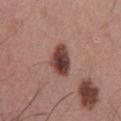Q: Is there a histopathology result?
A: catalogued during a skin exam; not biopsied
Q: What is the anatomic site?
A: the chest
Q: Patient demographics?
A: male, roughly 40 years of age
Q: What lighting was used for the tile?
A: white-light
Q: How was this image acquired?
A: ~15 mm tile from a whole-body skin photo
Q: Lesion size?
A: about 4 mm
Q: What did automated image analysis measure?
A: a mean CIELAB color near L≈41 a*≈21 b*≈23, about 16 CIELAB-L* units darker than the surrounding skin, and a lesion-to-skin contrast of about 12.5 (normalized; higher = more distinct); a peripheral color-asymmetry measure near 2